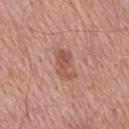Clinical impression: No biopsy was performed on this lesion — it was imaged during a full skin examination and was not determined to be concerning. Image and clinical context: The tile uses white-light illumination. A male patient, aged approximately 60. Located on the mid back. A roughly 15 mm field-of-view crop from a total-body skin photograph.